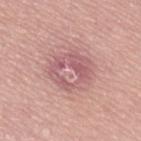Q: What is the lesion's diameter?
A: about 5 mm
Q: What is the anatomic site?
A: the lower back
Q: What kind of image is this?
A: total-body-photography crop, ~15 mm field of view
Q: Automated lesion metrics?
A: a peripheral color-asymmetry measure near 2.5; lesion-presence confidence of about 80/100
Q: Patient demographics?
A: male, in their 70s